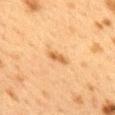  biopsy_status: not biopsied; imaged during a skin examination
  lighting: cross-polarized
  lesion_size:
    long_diameter_mm_approx: 3.0
  image:
    source: total-body photography crop
    field_of_view_mm: 15
  site: mid back
  patient:
    sex: female
    age_approx: 40